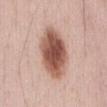biopsy_status: not biopsied; imaged during a skin examination
patient:
  sex: male
  age_approx: 50
image:
  source: total-body photography crop
  field_of_view_mm: 15
automated_metrics:
  vs_skin_darker_L: 18.0
  vs_skin_contrast_norm: 11.5
  lesion_detection_confidence_0_100: 100
lighting: white-light
lesion_size:
  long_diameter_mm_approx: 7.0
site: abdomen